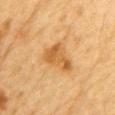| feature | finding |
|---|---|
| workup | no biopsy performed (imaged during a skin exam) |
| size | ≈4.5 mm |
| body site | the abdomen |
| patient | male, aged 83 or older |
| lighting | cross-polarized illumination |
| image source | 15 mm crop, total-body photography |